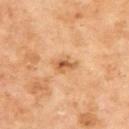Impression: This lesion was catalogued during total-body skin photography and was not selected for biopsy. Background: Captured under cross-polarized illumination. A 15 mm close-up tile from a total-body photography series done for melanoma screening. From the back. About 3 mm across. A male patient about 70 years old.